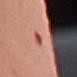Assessment: Imaged during a routine full-body skin examination; the lesion was not biopsied and no histopathology is available. Background: This image is a 15 mm lesion crop taken from a total-body photograph. A female subject, aged 63 to 67. Captured under white-light illumination. The lesion is on the right upper arm.